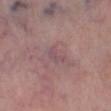Part of a total-body skin-imaging series; this lesion was reviewed on a skin check and was not flagged for biopsy.
Located on the left lower leg.
A male patient aged approximately 55.
Cropped from a whole-body photographic skin survey; the tile spans about 15 mm.